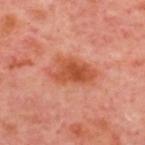Impression:
The lesion was photographed on a routine skin check and not biopsied; there is no pathology result.
Acquisition and patient details:
A patient aged approximately 55. Cropped from a whole-body photographic skin survey; the tile spans about 15 mm. Located on the upper back. Longest diameter approximately 6 mm.